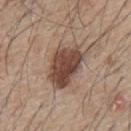This lesion was catalogued during total-body skin photography and was not selected for biopsy. The tile uses white-light illumination. Automated image analysis of the tile measured a footprint of about 16 mm², a shape eccentricity near 0.8, and two-axis asymmetry of about 0.25. It also reported an average lesion color of about L≈45 a*≈18 b*≈25 (CIELAB), about 15 CIELAB-L* units darker than the surrounding skin, and a normalized lesion–skin contrast near 11. The analysis additionally found border irregularity of about 3 on a 0–10 scale, a within-lesion color-variation index near 4.5/10, and a peripheral color-asymmetry measure near 1.5. It also reported a classifier nevus-likeness of about 75/100. The recorded lesion diameter is about 6 mm. The lesion is on the mid back. A male subject, approximately 65 years of age. Cropped from a total-body skin-imaging series; the visible field is about 15 mm.